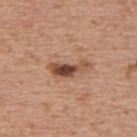The lesion was photographed on a routine skin check and not biopsied; there is no pathology result. A close-up tile cropped from a whole-body skin photograph, about 15 mm across. The lesion is located on the upper back. Captured under white-light illumination. The lesion's longest dimension is about 5 mm. A male subject, roughly 70 years of age. An algorithmic analysis of the crop reported an area of roughly 7 mm², an outline eccentricity of about 0.95 (0 = round, 1 = elongated), and a symmetry-axis asymmetry near 0.35. And it measured a mean CIELAB color near L≈48 a*≈22 b*≈30, about 14 CIELAB-L* units darker than the surrounding skin, and a lesion-to-skin contrast of about 10 (normalized; higher = more distinct).